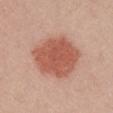imaging modality — ~15 mm crop, total-body skin-cancer survey
automated metrics — a lesion area of about 26 mm², an eccentricity of roughly 0.5, and a shape-asymmetry score of about 0.15 (0 = symmetric); a mean CIELAB color near L≈56 a*≈27 b*≈30, a lesion–skin lightness drop of about 12, and a normalized border contrast of about 8.5; border irregularity of about 1.5 on a 0–10 scale and radial color variation of about 0.5; a classifier nevus-likeness of about 100/100
body site — the chest
lesion size — ~6.5 mm (longest diameter)
patient — female, in their mid-30s